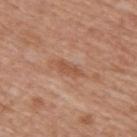Notes:
* workup: no biopsy performed (imaged during a skin exam)
* patient: male, roughly 65 years of age
* imaging modality: 15 mm crop, total-body photography
* illumination: white-light illumination
* lesion size: ~3 mm (longest diameter)
* automated metrics: a lesion color around L≈52 a*≈23 b*≈32 in CIELAB, roughly 8 lightness units darker than nearby skin, and a normalized border contrast of about 6; a lesion-detection confidence of about 100/100
* body site: the upper back